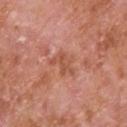No biopsy was performed on this lesion — it was imaged during a full skin examination and was not determined to be concerning.
About 3.5 mm across.
An algorithmic analysis of the crop reported a lesion area of about 6 mm², an eccentricity of roughly 0.7, and a symmetry-axis asymmetry near 0.4. The analysis additionally found an average lesion color of about L≈54 a*≈27 b*≈32 (CIELAB) and a normalized lesion–skin contrast near 6. The software also gave border irregularity of about 5 on a 0–10 scale and internal color variation of about 3 on a 0–10 scale. The software also gave an automated nevus-likeness rating near 0 out of 100 and a lesion-detection confidence of about 100/100.
The patient is a male aged 63–67.
Imaged with white-light lighting.
A 15 mm crop from a total-body photograph taken for skin-cancer surveillance.
Located on the upper back.